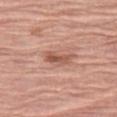  biopsy_status: not biopsied; imaged during a skin examination
  site: left thigh
  lesion_size:
    long_diameter_mm_approx: 3.5
  image:
    source: total-body photography crop
    field_of_view_mm: 15
  lighting: white-light
  patient:
    sex: female
    age_approx: 75